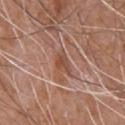Clinical impression:
Captured during whole-body skin photography for melanoma surveillance; the lesion was not biopsied.
Acquisition and patient details:
The lesion is on the back. Longest diameter approximately 2.5 mm. A 15 mm close-up tile from a total-body photography series done for melanoma screening. The total-body-photography lesion software estimated an automated nevus-likeness rating near 0 out of 100 and a detector confidence of about 90 out of 100 that the crop contains a lesion. The tile uses white-light illumination. A male subject aged approximately 60.